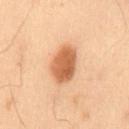This lesion was catalogued during total-body skin photography and was not selected for biopsy.
The recorded lesion diameter is about 5 mm.
The lesion is on the back.
A lesion tile, about 15 mm wide, cut from a 3D total-body photograph.
A male subject, roughly 55 years of age.
This is a cross-polarized tile.